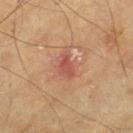Impression:
This lesion was catalogued during total-body skin photography and was not selected for biopsy.
Clinical summary:
This is a cross-polarized tile. A region of skin cropped from a whole-body photographic capture, roughly 15 mm wide. The recorded lesion diameter is about 2.5 mm. A male subject, approximately 70 years of age. From the left thigh.